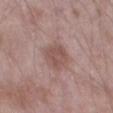follow-up: no biopsy performed (imaged during a skin exam); illumination: white-light illumination; subject: male, aged 48–52; lesion size: ~3.5 mm (longest diameter); image source: ~15 mm crop, total-body skin-cancer survey; anatomic site: the left thigh.